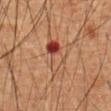Q: How was this image acquired?
A: total-body-photography crop, ~15 mm field of view
Q: What did automated image analysis measure?
A: an average lesion color of about L≈43 a*≈24 b*≈29 (CIELAB), roughly 9 lightness units darker than nearby skin, and a normalized lesion–skin contrast near 7.5; a border-irregularity index near 4/10, internal color variation of about 10 on a 0–10 scale, and peripheral color asymmetry of about 7
Q: Where on the body is the lesion?
A: the mid back
Q: How large is the lesion?
A: ~4 mm (longest diameter)
Q: Illumination type?
A: cross-polarized
Q: What are the patient's age and sex?
A: male, in their 60s